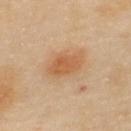This lesion was catalogued during total-body skin photography and was not selected for biopsy. The subject is a female in their 40s. On the upper back. This image is a 15 mm lesion crop taken from a total-body photograph. Captured under cross-polarized illumination. The recorded lesion diameter is about 4 mm.